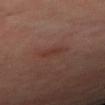| field | value |
|---|---|
| follow-up | imaged on a skin check; not biopsied |
| subject | female |
| location | the left thigh |
| size | ~3 mm (longest diameter) |
| acquisition | 15 mm crop, total-body photography |